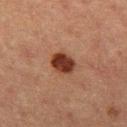{
  "biopsy_status": "not biopsied; imaged during a skin examination",
  "patient": {
    "sex": "female",
    "age_approx": 40
  },
  "image": {
    "source": "total-body photography crop",
    "field_of_view_mm": 15
  },
  "site": "left thigh"
}